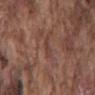Imaged during a routine full-body skin examination; the lesion was not biopsied and no histopathology is available.